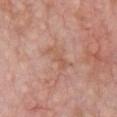follow-up: no biopsy performed (imaged during a skin exam)
automated lesion analysis: a nevus-likeness score of about 0/100 and a detector confidence of about 100 out of 100 that the crop contains a lesion
anatomic site: the chest
imaging modality: ~15 mm crop, total-body skin-cancer survey
illumination: white-light
subject: male, aged around 60
lesion size: ~3.5 mm (longest diameter)